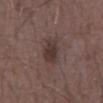workup = imaged on a skin check; not biopsied
subject = male, aged 58 to 62
image = total-body-photography crop, ~15 mm field of view
anatomic site = the chest